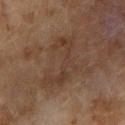Background: A female subject, approximately 60 years of age. Imaged with cross-polarized lighting. A lesion tile, about 15 mm wide, cut from a 3D total-body photograph. Measured at roughly 8 mm in maximum diameter. Located on the right upper arm.